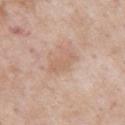biopsy status: no biopsy performed (imaged during a skin exam)
body site: the left upper arm
image: ~15 mm tile from a whole-body skin photo
size: ≈3.5 mm
subject: male, in their mid- to late 50s
image-analysis metrics: border irregularity of about 3 on a 0–10 scale, internal color variation of about 1.5 on a 0–10 scale, and radial color variation of about 0.5; a nevus-likeness score of about 0/100 and lesion-presence confidence of about 100/100
tile lighting: white-light illumination